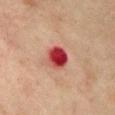Assessment:
Recorded during total-body skin imaging; not selected for excision or biopsy.
Background:
This is a cross-polarized tile. Located on the front of the torso. A female patient aged 68 to 72. Longest diameter approximately 3 mm. A lesion tile, about 15 mm wide, cut from a 3D total-body photograph. An algorithmic analysis of the crop reported an area of roughly 7 mm² and a shape eccentricity near 0.45. The analysis additionally found an automated nevus-likeness rating near 0 out of 100 and a lesion-detection confidence of about 100/100.